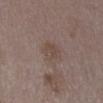workup=catalogued during a skin exam; not biopsied
subject=female, aged approximately 35
site=the abdomen
illumination=white-light
image=~15 mm crop, total-body skin-cancer survey
automated lesion analysis=a mean CIELAB color near L≈46 a*≈14 b*≈22 and roughly 6 lightness units darker than nearby skin; a border-irregularity index near 2/10, a color-variation rating of about 2/10, and radial color variation of about 0.5; a detector confidence of about 100 out of 100 that the crop contains a lesion